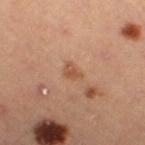biopsy status — catalogued during a skin exam; not biopsied
lesion diameter — ~2.5 mm (longest diameter)
imaging modality — total-body-photography crop, ~15 mm field of view
anatomic site — the right thigh
patient — female, aged around 45
tile lighting — cross-polarized illumination